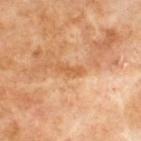workup = imaged on a skin check; not biopsied
patient = male, about 70 years old
body site = the upper back
acquisition = total-body-photography crop, ~15 mm field of view